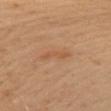biopsy status: catalogued during a skin exam; not biopsied | image: total-body-photography crop, ~15 mm field of view | patient: male, roughly 55 years of age | automated lesion analysis: a lesion color around L≈53 a*≈21 b*≈35 in CIELAB, a lesion–skin lightness drop of about 6, and a normalized lesion–skin contrast near 4.5 | body site: the left upper arm | lighting: cross-polarized illumination.